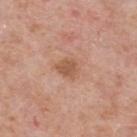Q: Is there a histopathology result?
A: imaged on a skin check; not biopsied
Q: Lesion size?
A: about 3 mm
Q: What is the imaging modality?
A: 15 mm crop, total-body photography
Q: Where on the body is the lesion?
A: the upper back
Q: How was the tile lit?
A: white-light illumination
Q: What are the patient's age and sex?
A: male, aged 73–77